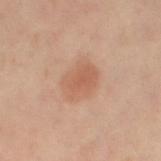Case summary:
• workup · total-body-photography surveillance lesion; no biopsy
• location · the left thigh
• subject · female, aged 48–52
• imaging modality · ~15 mm tile from a whole-body skin photo